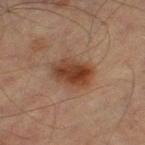Image and clinical context:
The lesion is on the left thigh. This is a cross-polarized tile. An algorithmic analysis of the crop reported an area of roughly 11 mm², an eccentricity of roughly 0.75, and a shape-asymmetry score of about 0.15 (0 = symmetric). And it measured an average lesion color of about L≈34 a*≈19 b*≈26 (CIELAB) and roughly 10 lightness units darker than nearby skin. It also reported a border-irregularity index near 2/10 and a color-variation rating of about 5/10. The software also gave a classifier nevus-likeness of about 95/100 and a detector confidence of about 100 out of 100 that the crop contains a lesion. Measured at roughly 4.5 mm in maximum diameter. Cropped from a whole-body photographic skin survey; the tile spans about 15 mm. The patient is a male approximately 75 years of age.